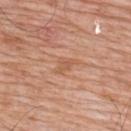Assessment:
The lesion was photographed on a routine skin check and not biopsied; there is no pathology result.
Background:
The patient is a male aged 78 to 82. On the upper back. A close-up tile cropped from a whole-body skin photograph, about 15 mm across. About 3.5 mm across. The tile uses white-light illumination.